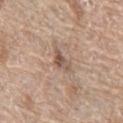Impression: Recorded during total-body skin imaging; not selected for excision or biopsy. Background: On the left thigh. This image is a 15 mm lesion crop taken from a total-body photograph. A female patient, in their 60s.